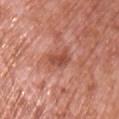{
  "biopsy_status": "not biopsied; imaged during a skin examination",
  "automated_metrics": {
    "eccentricity": 0.85,
    "cielab_L": 50,
    "cielab_a": 28,
    "cielab_b": 31,
    "vs_skin_darker_L": 10.0,
    "nevus_likeness_0_100": 0,
    "lesion_detection_confidence_0_100": 100
  },
  "image": {
    "source": "total-body photography crop",
    "field_of_view_mm": 15
  },
  "lighting": "white-light",
  "patient": {
    "sex": "male",
    "age_approx": 70
  },
  "site": "chest"
}